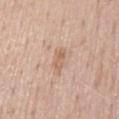Assessment:
Recorded during total-body skin imaging; not selected for excision or biopsy.
Clinical summary:
A male patient, about 50 years old. The tile uses white-light illumination. The lesion's longest dimension is about 2.5 mm. A 15 mm close-up extracted from a 3D total-body photography capture. From the back. Automated tile analysis of the lesion measured a footprint of about 3 mm², a shape eccentricity near 0.9, and a symmetry-axis asymmetry near 0.2. The software also gave a lesion–skin lightness drop of about 8. The analysis additionally found a color-variation rating of about 2.5/10 and peripheral color asymmetry of about 1. The analysis additionally found a classifier nevus-likeness of about 0/100 and lesion-presence confidence of about 100/100.